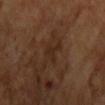Clinical impression:
No biopsy was performed on this lesion — it was imaged during a full skin examination and was not determined to be concerning.
Clinical summary:
A male patient, roughly 65 years of age. Automated image analysis of the tile measured an eccentricity of roughly 0.8 and a shape-asymmetry score of about 0.65 (0 = symmetric). The software also gave a lesion color around L≈24 a*≈17 b*≈25 in CIELAB, a lesion–skin lightness drop of about 5, and a normalized lesion–skin contrast near 6. And it measured a border-irregularity rating of about 7/10, internal color variation of about 1 on a 0–10 scale, and a peripheral color-asymmetry measure near 0.5. The software also gave a nevus-likeness score of about 0/100 and a detector confidence of about 100 out of 100 that the crop contains a lesion. A roughly 15 mm field-of-view crop from a total-body skin photograph. Imaged with cross-polarized lighting. Located on the front of the torso.